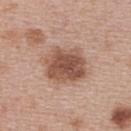biopsy status: total-body-photography surveillance lesion; no biopsy
TBP lesion metrics: an eccentricity of roughly 0.65 and a shape-asymmetry score of about 0.2 (0 = symmetric)
size: ~5 mm (longest diameter)
illumination: white-light
patient: female, aged around 50
image: ~15 mm crop, total-body skin-cancer survey
site: the upper back